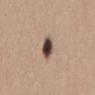Case summary:
– workup · catalogued during a skin exam; not biopsied
– acquisition · 15 mm crop, total-body photography
– tile lighting · white-light illumination
– lesion diameter · ≈2.5 mm
– patient · female, aged approximately 40
– body site · the lower back